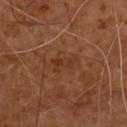Q: Was a biopsy performed?
A: catalogued during a skin exam; not biopsied
Q: Lesion location?
A: the chest
Q: Patient demographics?
A: male, in their mid- to late 50s
Q: Lesion size?
A: ~3 mm (longest diameter)
Q: How was this image acquired?
A: total-body-photography crop, ~15 mm field of view
Q: Automated lesion metrics?
A: a lesion area of about 4 mm², a shape eccentricity near 0.85, and a symmetry-axis asymmetry near 0.4; a border-irregularity rating of about 5/10, a color-variation rating of about 2.5/10, and radial color variation of about 0.5; a nevus-likeness score of about 0/100
Q: What lighting was used for the tile?
A: cross-polarized